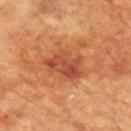biopsy status: no biopsy performed (imaged during a skin exam); image source: ~15 mm crop, total-body skin-cancer survey; body site: the arm; patient: male, in their mid- to late 70s; lesion diameter: ≈5 mm; tile lighting: cross-polarized.